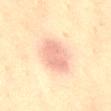A 15 mm close-up extracted from a 3D total-body photography capture.
The lesion's longest dimension is about 5 mm.
The total-body-photography lesion software estimated a footprint of about 13 mm² and a shape eccentricity near 0.65. The analysis additionally found a mean CIELAB color near L≈66 a*≈19 b*≈28, a lesion–skin lightness drop of about 10, and a lesion-to-skin contrast of about 6.5 (normalized; higher = more distinct).
The tile uses cross-polarized illumination.
A female patient in their 80s.
Located on the abdomen.